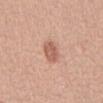Recorded during total-body skin imaging; not selected for excision or biopsy.
Automated image analysis of the tile measured roughly 11 lightness units darker than nearby skin and a lesion-to-skin contrast of about 7 (normalized; higher = more distinct). The analysis additionally found a color-variation rating of about 2.5/10.
A female subject in their mid-50s.
The tile uses white-light illumination.
The lesion is located on the front of the torso.
A 15 mm crop from a total-body photograph taken for skin-cancer surveillance.
Longest diameter approximately 3 mm.